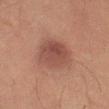Clinical impression:
Part of a total-body skin-imaging series; this lesion was reviewed on a skin check and was not flagged for biopsy.
Context:
A male subject aged approximately 70. A 15 mm close-up extracted from a 3D total-body photography capture. Captured under cross-polarized illumination. The lesion is located on the right thigh.